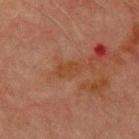<tbp_lesion>
  <biopsy_status>not biopsied; imaged during a skin examination</biopsy_status>
  <lighting>cross-polarized</lighting>
  <patient>
    <sex>male</sex>
    <age_approx>70</age_approx>
  </patient>
  <site>chest</site>
  <image>
    <source>total-body photography crop</source>
    <field_of_view_mm>15</field_of_view_mm>
  </image>
  <lesion_size>
    <long_diameter_mm_approx>3.5</long_diameter_mm_approx>
  </lesion_size>
</tbp_lesion>